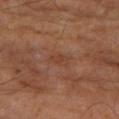The lesion was tiled from a total-body skin photograph and was not biopsied.
A male subject, aged 63 to 67.
A 15 mm close-up extracted from a 3D total-body photography capture.